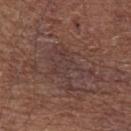Q: Was a biopsy performed?
A: catalogued during a skin exam; not biopsied
Q: Where on the body is the lesion?
A: the left forearm
Q: What lighting was used for the tile?
A: white-light illumination
Q: What is the lesion's diameter?
A: ≈5.5 mm
Q: What are the patient's age and sex?
A: male, aged approximately 65
Q: What is the imaging modality?
A: total-body-photography crop, ~15 mm field of view